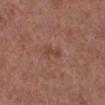| field | value |
|---|---|
| follow-up | imaged on a skin check; not biopsied |